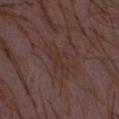Impression:
Captured during whole-body skin photography for melanoma surveillance; the lesion was not biopsied.
Image and clinical context:
A female subject, aged 28–32. The tile uses white-light illumination. Automated tile analysis of the lesion measured a footprint of about 8 mm², a shape eccentricity near 0.9, and a shape-asymmetry score of about 0.35 (0 = symmetric). And it measured a mean CIELAB color near L≈32 a*≈17 b*≈22, about 3 CIELAB-L* units darker than the surrounding skin, and a lesion-to-skin contrast of about 5 (normalized; higher = more distinct). The software also gave a nevus-likeness score of about 0/100 and a detector confidence of about 90 out of 100 that the crop contains a lesion. Located on the abdomen. A 15 mm crop from a total-body photograph taken for skin-cancer surveillance.